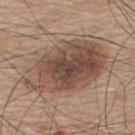This lesion was catalogued during total-body skin photography and was not selected for biopsy.
A male patient, roughly 75 years of age.
A region of skin cropped from a whole-body photographic capture, roughly 15 mm wide.
The lesion is located on the upper back.
This is a white-light tile.